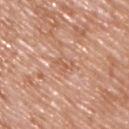biopsy_status: not biopsied; imaged during a skin examination
patient:
  sex: male
  age_approx: 50
site: back
lighting: white-light
lesion_size:
  long_diameter_mm_approx: 2.5
image:
  source: total-body photography crop
  field_of_view_mm: 15